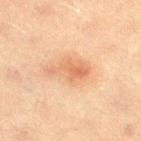Impression: This lesion was catalogued during total-body skin photography and was not selected for biopsy. Image and clinical context: The lesion's longest dimension is about 5 mm. From the right thigh. This is a cross-polarized tile. A 15 mm crop from a total-body photograph taken for skin-cancer surveillance. The total-body-photography lesion software estimated a lesion area of about 9.5 mm² and two-axis asymmetry of about 0.4. And it measured a lesion color around L≈55 a*≈20 b*≈32 in CIELAB, about 8 CIELAB-L* units darker than the surrounding skin, and a normalized lesion–skin contrast near 6. It also reported border irregularity of about 4 on a 0–10 scale, internal color variation of about 4 on a 0–10 scale, and a peripheral color-asymmetry measure near 1.5. The analysis additionally found a nevus-likeness score of about 95/100 and a detector confidence of about 100 out of 100 that the crop contains a lesion. A male patient, about 40 years old.